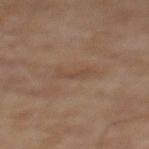Part of a total-body skin-imaging series; this lesion was reviewed on a skin check and was not flagged for biopsy. Captured under cross-polarized illumination. Located on the mid back. A male subject, aged approximately 65. Automated image analysis of the tile measured a mean CIELAB color near L≈43 a*≈16 b*≈26, about 5 CIELAB-L* units darker than the surrounding skin, and a normalized lesion–skin contrast near 4.5. It also reported a nevus-likeness score of about 0/100 and lesion-presence confidence of about 95/100. A 15 mm close-up extracted from a 3D total-body photography capture.